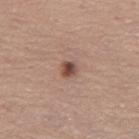– automated lesion analysis: an area of roughly 3 mm², an outline eccentricity of about 0.45 (0 = round, 1 = elongated), and a symmetry-axis asymmetry near 0.2; an average lesion color of about L≈48 a*≈20 b*≈25 (CIELAB), roughly 13 lightness units darker than nearby skin, and a normalized lesion–skin contrast near 9.5; a border-irregularity rating of about 1.5/10, a within-lesion color-variation index near 5/10, and radial color variation of about 2; a classifier nevus-likeness of about 90/100 and a lesion-detection confidence of about 100/100
– lighting: white-light illumination
– location: the leg
– subject: female, about 65 years old
– diameter: about 2 mm
– acquisition: ~15 mm crop, total-body skin-cancer survey